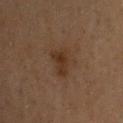Part of a total-body skin-imaging series; this lesion was reviewed on a skin check and was not flagged for biopsy. This image is a 15 mm lesion crop taken from a total-body photograph. The lesion's longest dimension is about 3 mm. Captured under cross-polarized illumination. An algorithmic analysis of the crop reported a mean CIELAB color near L≈28 a*≈15 b*≈25, about 6 CIELAB-L* units darker than the surrounding skin, and a normalized border contrast of about 7. The software also gave a border-irregularity rating of about 3.5/10, a color-variation rating of about 3/10, and radial color variation of about 1. A male subject approximately 60 years of age.